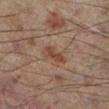Acquisition and patient details: This image is a 15 mm lesion crop taken from a total-body photograph. On the left lower leg. This is a cross-polarized tile. A male subject, roughly 60 years of age. The total-body-photography lesion software estimated a footprint of about 4.5 mm² and an outline eccentricity of about 0.85 (0 = round, 1 = elongated).